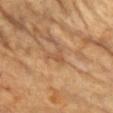Notes:
- follow-up — imaged on a skin check; not biopsied
- lighting — cross-polarized
- lesion diameter — ~2.5 mm (longest diameter)
- site — the chest
- patient — female, aged 58–62
- imaging modality — total-body-photography crop, ~15 mm field of view
- TBP lesion metrics — a lesion color around L≈55 a*≈21 b*≈36 in CIELAB, about 7 CIELAB-L* units darker than the surrounding skin, and a normalized border contrast of about 5; a border-irregularity rating of about 6/10, internal color variation of about 1.5 on a 0–10 scale, and peripheral color asymmetry of about 0.5; a classifier nevus-likeness of about 0/100 and a detector confidence of about 80 out of 100 that the crop contains a lesion